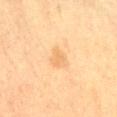workup = no biopsy performed (imaged during a skin exam) | imaging modality = total-body-photography crop, ~15 mm field of view | subject = female, in their 40s | site = the lower back.